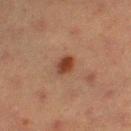No biopsy was performed on this lesion — it was imaged during a full skin examination and was not determined to be concerning.
A lesion tile, about 15 mm wide, cut from a 3D total-body photograph.
About 2.5 mm across.
From the leg.
A female subject roughly 55 years of age.